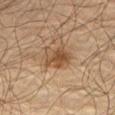Notes:
• biopsy status · catalogued during a skin exam; not biopsied
• site · the abdomen
• patient · male, aged around 70
• image · ~15 mm crop, total-body skin-cancer survey
• tile lighting · cross-polarized
• lesion diameter · ~3.5 mm (longest diameter)